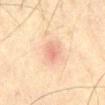Recorded during total-body skin imaging; not selected for excision or biopsy. The lesion-visualizer software estimated a border-irregularity index near 2.5/10 and a within-lesion color-variation index near 2/10. The software also gave a nevus-likeness score of about 0/100 and a detector confidence of about 100 out of 100 that the crop contains a lesion. About 2.5 mm across. The lesion is on the abdomen. A lesion tile, about 15 mm wide, cut from a 3D total-body photograph. This is a cross-polarized tile. The subject is a male in their mid- to late 70s.